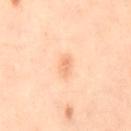workup: total-body-photography surveillance lesion; no biopsy
lighting: cross-polarized
automated lesion analysis: a lesion area of about 3.5 mm²; border irregularity of about 2.5 on a 0–10 scale, a color-variation rating of about 2/10, and peripheral color asymmetry of about 0.5
patient: female, aged approximately 40
site: the leg
lesion size: ≈2.5 mm
image source: 15 mm crop, total-body photography